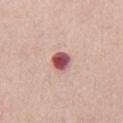Assessment: No biopsy was performed on this lesion — it was imaged during a full skin examination and was not determined to be concerning. Clinical summary: A male patient aged around 50. A region of skin cropped from a whole-body photographic capture, roughly 15 mm wide. Imaged with white-light lighting. From the front of the torso. Longest diameter approximately 2.5 mm.